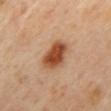* biopsy status — no biopsy performed (imaged during a skin exam)
* imaging modality — ~15 mm crop, total-body skin-cancer survey
* patient — male, aged around 55
* location — the mid back
* lesion diameter — ~4 mm (longest diameter)
* lighting — cross-polarized